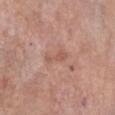{
  "biopsy_status": "not biopsied; imaged during a skin examination",
  "image": {
    "source": "total-body photography crop",
    "field_of_view_mm": 15
  },
  "site": "chest",
  "automated_metrics": {
    "border_irregularity_0_10": 4.0,
    "color_variation_0_10": 0.5,
    "peripheral_color_asymmetry": 0.0,
    "nevus_likeness_0_100": 0,
    "lesion_detection_confidence_0_100": 100
  },
  "patient": {
    "sex": "female",
    "age_approx": 65
  },
  "lesion_size": {
    "long_diameter_mm_approx": 3.0
  }
}